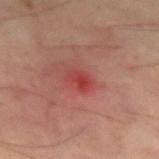The lesion was photographed on a routine skin check and not biopsied; there is no pathology result.
The patient is a male roughly 60 years of age.
The lesion is on the left thigh.
Cropped from a whole-body photographic skin survey; the tile spans about 15 mm.